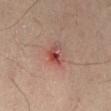workup: catalogued during a skin exam; not biopsied | subject: male, approximately 50 years of age | size: ~2.5 mm (longest diameter) | lighting: cross-polarized | imaging modality: 15 mm crop, total-body photography | location: the lower back | automated metrics: a mean CIELAB color near L≈42 a*≈25 b*≈23.